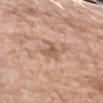Part of a total-body skin-imaging series; this lesion was reviewed on a skin check and was not flagged for biopsy. About 3 mm across. This is a white-light tile. Automated tile analysis of the lesion measured an area of roughly 4 mm² and a shape-asymmetry score of about 0.4 (0 = symmetric). It also reported an average lesion color of about L≈58 a*≈21 b*≈29 (CIELAB), about 9 CIELAB-L* units darker than the surrounding skin, and a lesion-to-skin contrast of about 6 (normalized; higher = more distinct). A female subject, aged around 70. From the left forearm. This image is a 15 mm lesion crop taken from a total-body photograph.